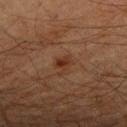The lesion was photographed on a routine skin check and not biopsied; there is no pathology result.
The patient is a male aged around 65.
Captured under cross-polarized illumination.
Longest diameter approximately 2.5 mm.
The lesion is located on the right forearm.
A region of skin cropped from a whole-body photographic capture, roughly 15 mm wide.